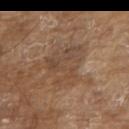Q: Was this lesion biopsied?
A: no biopsy performed (imaged during a skin exam)
Q: Where on the body is the lesion?
A: the upper back
Q: What is the lesion's diameter?
A: ~5 mm (longest diameter)
Q: Patient demographics?
A: male, aged 78–82
Q: What kind of image is this?
A: ~15 mm crop, total-body skin-cancer survey